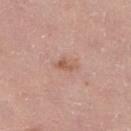Q: Where on the body is the lesion?
A: the right lower leg
Q: What are the patient's age and sex?
A: female, aged approximately 65
Q: What is the imaging modality?
A: total-body-photography crop, ~15 mm field of view
Q: What is the lesion's diameter?
A: ≈2.5 mm
Q: How was the tile lit?
A: white-light illumination